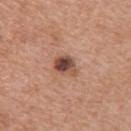Findings:
– biopsy status · total-body-photography surveillance lesion; no biopsy
– subject · female, in their mid-50s
– body site · the back
– image · ~15 mm tile from a whole-body skin photo